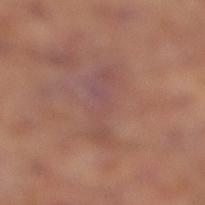lesion diameter=~6.5 mm (longest diameter)
site=the right lower leg
automated metrics=an area of roughly 11 mm², a shape eccentricity near 0.95, and two-axis asymmetry of about 0.35; a color-variation rating of about 3/10 and radial color variation of about 1; a nevus-likeness score of about 0/100
patient=male, in their mid-60s
image source=~15 mm crop, total-body skin-cancer survey